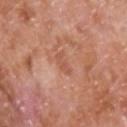Case summary:
* notes · no biopsy performed (imaged during a skin exam)
* imaging modality · total-body-photography crop, ~15 mm field of view
* image-analysis metrics · a footprint of about 3 mm² and two-axis asymmetry of about 0.3; a classifier nevus-likeness of about 0/100 and a lesion-detection confidence of about 100/100
* size · ~2.5 mm (longest diameter)
* body site · the chest
* illumination · white-light
* patient · male, roughly 65 years of age